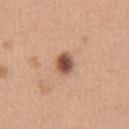Clinical impression: Imaged during a routine full-body skin examination; the lesion was not biopsied and no histopathology is available. Acquisition and patient details: The lesion is located on the abdomen. A lesion tile, about 15 mm wide, cut from a 3D total-body photograph. The total-body-photography lesion software estimated a detector confidence of about 100 out of 100 that the crop contains a lesion. This is a white-light tile. A male subject approximately 40 years of age.